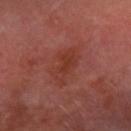workup: imaged on a skin check; not biopsied | site: the right forearm | image: ~15 mm crop, total-body skin-cancer survey | subject: male, aged around 70 | tile lighting: cross-polarized illumination | image-analysis metrics: a footprint of about 9 mm² and two-axis asymmetry of about 0.35; a mean CIELAB color near L≈36 a*≈27 b*≈27, a lesion–skin lightness drop of about 6, and a lesion-to-skin contrast of about 6 (normalized; higher = more distinct); a border-irregularity rating of about 4/10 | size: ≈5 mm.